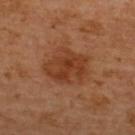No biopsy was performed on this lesion — it was imaged during a full skin examination and was not determined to be concerning.
The subject is a female roughly 55 years of age.
Longest diameter approximately 5.5 mm.
The total-body-photography lesion software estimated a footprint of about 18 mm², an outline eccentricity of about 0.6 (0 = round, 1 = elongated), and a symmetry-axis asymmetry near 0.25. It also reported border irregularity of about 2.5 on a 0–10 scale and a color-variation rating of about 4.5/10.
This image is a 15 mm lesion crop taken from a total-body photograph.
Located on the upper back.